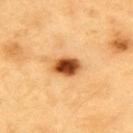follow-up = catalogued during a skin exam; not biopsied | illumination = cross-polarized illumination | lesion size = about 3.5 mm | acquisition = 15 mm crop, total-body photography | location = the upper back | automated lesion analysis = a mean CIELAB color near L≈52 a*≈27 b*≈44 and roughly 22 lightness units darker than nearby skin; an automated nevus-likeness rating near 100 out of 100 and a lesion-detection confidence of about 100/100 | subject = male, aged approximately 60.